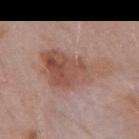follow-up: catalogued during a skin exam; not biopsied | illumination: white-light | diameter: ≈7.5 mm | TBP lesion metrics: an automated nevus-likeness rating near 70 out of 100 and lesion-presence confidence of about 100/100 | patient: male, in their mid- to late 50s | image source: 15 mm crop, total-body photography | location: the mid back.